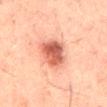<lesion>
<biopsy_status>not biopsied; imaged during a skin examination</biopsy_status>
<lesion_size>
  <long_diameter_mm_approx>5.0</long_diameter_mm_approx>
</lesion_size>
<patient>
  <sex>male</sex>
  <age_approx>50</age_approx>
</patient>
<site>mid back</site>
<automated_metrics>
  <cielab_L>48</cielab_L>
  <cielab_a>25</cielab_a>
  <cielab_b>27</cielab_b>
  <vs_skin_darker_L>13.0</vs_skin_darker_L>
  <vs_skin_contrast_norm>9.5</vs_skin_contrast_norm>
  <nevus_likeness_0_100>95</nevus_likeness_0_100>
  <lesion_detection_confidence_0_100>100</lesion_detection_confidence_0_100>
</automated_metrics>
<image>
  <source>total-body photography crop</source>
  <field_of_view_mm>15</field_of_view_mm>
</image>
</lesion>